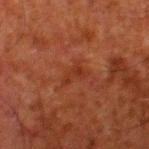Q: Was a biopsy performed?
A: catalogued during a skin exam; not biopsied
Q: How large is the lesion?
A: ~3 mm (longest diameter)
Q: Patient demographics?
A: male, in their 80s
Q: Automated lesion metrics?
A: about 5 CIELAB-L* units darker than the surrounding skin and a normalized border contrast of about 5.5; border irregularity of about 7 on a 0–10 scale, a within-lesion color-variation index near 0/10, and a peripheral color-asymmetry measure near 0; lesion-presence confidence of about 100/100
Q: What is the imaging modality?
A: ~15 mm tile from a whole-body skin photo
Q: How was the tile lit?
A: cross-polarized illumination
Q: What is the anatomic site?
A: the leg